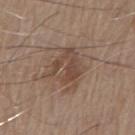The lesion is located on the chest. The patient is a male in their 80s. A close-up tile cropped from a whole-body skin photograph, about 15 mm across. The lesion-visualizer software estimated an average lesion color of about L≈45 a*≈16 b*≈26 (CIELAB) and a lesion–skin lightness drop of about 8. The software also gave a within-lesion color-variation index near 2.5/10. Imaged with white-light lighting. About 4.5 mm across.